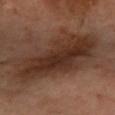workup: imaged on a skin check; not biopsied
lesion size: about 10.5 mm
illumination: cross-polarized illumination
location: the arm
image: total-body-photography crop, ~15 mm field of view
subject: female, aged around 60